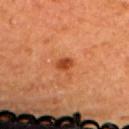Assessment: This lesion was catalogued during total-body skin photography and was not selected for biopsy. Acquisition and patient details: Imaged with cross-polarized lighting. Automated image analysis of the tile measured a border-irregularity rating of about 1.5/10 and a within-lesion color-variation index near 2/10. A region of skin cropped from a whole-body photographic capture, roughly 15 mm wide. The lesion is on the back. The subject is a female in their mid-60s. The lesion's longest dimension is about 2 mm.